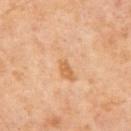Case summary:
* notes · total-body-photography surveillance lesion; no biopsy
* image · total-body-photography crop, ~15 mm field of view
* patient · male, in their mid-60s
* size · about 5.5 mm